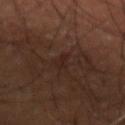Case summary:
- follow-up — no biopsy performed (imaged during a skin exam)
- imaging modality — ~15 mm tile from a whole-body skin photo
- patient — male, in their mid-50s
- anatomic site — the arm